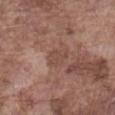Captured during whole-body skin photography for melanoma surveillance; the lesion was not biopsied. Imaged with white-light lighting. The patient is a male roughly 75 years of age. About 3 mm across. The lesion-visualizer software estimated a lesion area of about 5 mm² and a symmetry-axis asymmetry near 0.25. The analysis additionally found a border-irregularity index near 2.5/10 and a within-lesion color-variation index near 1.5/10. On the abdomen. A 15 mm close-up tile from a total-body photography series done for melanoma screening.